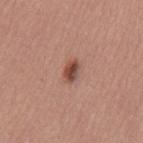Captured during whole-body skin photography for melanoma surveillance; the lesion was not biopsied. On the back. Automated image analysis of the tile measured a lesion area of about 3.5 mm² and two-axis asymmetry of about 0.25. The analysis additionally found a border-irregularity rating of about 2.5/10 and internal color variation of about 4.5 on a 0–10 scale. It also reported an automated nevus-likeness rating near 95 out of 100 and a lesion-detection confidence of about 100/100. A 15 mm close-up extracted from a 3D total-body photography capture. A female patient aged approximately 50.